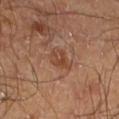Impression:
Captured during whole-body skin photography for melanoma surveillance; the lesion was not biopsied.
Acquisition and patient details:
A 15 mm close-up tile from a total-body photography series done for melanoma screening. Approximately 3 mm at its widest. The subject is a male aged around 60. The lesion is on the left lower leg.